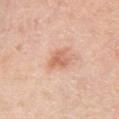Part of a total-body skin-imaging series; this lesion was reviewed on a skin check and was not flagged for biopsy. Cropped from a total-body skin-imaging series; the visible field is about 15 mm. On the front of the torso. A male subject in their mid-30s. The lesion-visualizer software estimated an eccentricity of roughly 0.65 and a symmetry-axis asymmetry near 0.35. The software also gave a mean CIELAB color near L≈65 a*≈23 b*≈33, a lesion–skin lightness drop of about 10, and a normalized border contrast of about 6.5. The analysis additionally found border irregularity of about 3.5 on a 0–10 scale and a peripheral color-asymmetry measure near 1. It also reported a classifier nevus-likeness of about 70/100 and lesion-presence confidence of about 100/100.